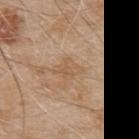  biopsy_status: not biopsied; imaged during a skin examination
  lesion_size:
    long_diameter_mm_approx: 2.5
  automated_metrics:
    area_mm2_approx: 3.5
    eccentricity: 0.65
    shape_asymmetry: 0.45
    cielab_L: 57
    cielab_a: 17
    cielab_b: 34
    vs_skin_darker_L: 6.0
    vs_skin_contrast_norm: 4.5
  patient:
    sex: male
    age_approx: 80
  lighting: white-light
  image:
    source: total-body photography crop
    field_of_view_mm: 15
  site: upper back